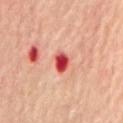The lesion was tiled from a total-body skin photograph and was not biopsied. On the mid back. Measured at roughly 2.5 mm in maximum diameter. A lesion tile, about 15 mm wide, cut from a 3D total-body photograph. A male subject aged 68–72.